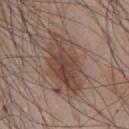Captured during whole-body skin photography for melanoma surveillance; the lesion was not biopsied.
A male subject in their mid- to late 40s.
Located on the chest.
Captured under white-light illumination.
Cropped from a total-body skin-imaging series; the visible field is about 15 mm.